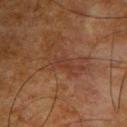biopsy status = catalogued during a skin exam; not biopsied
illumination = cross-polarized illumination
image source = total-body-photography crop, ~15 mm field of view
anatomic site = the upper back
lesion size = ~3 mm (longest diameter)
patient = male, approximately 65 years of age
TBP lesion metrics = an average lesion color of about L≈29 a*≈19 b*≈24 (CIELAB), about 4 CIELAB-L* units darker than the surrounding skin, and a normalized border contrast of about 4.5; a border-irregularity rating of about 4/10 and a peripheral color-asymmetry measure near 0; a lesion-detection confidence of about 55/100